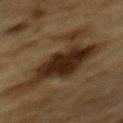Part of a total-body skin-imaging series; this lesion was reviewed on a skin check and was not flagged for biopsy. The lesion is on the back. A close-up tile cropped from a whole-body skin photograph, about 15 mm across. The subject is a male in their mid- to late 80s or older.